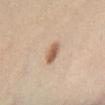Q: Is there a histopathology result?
A: imaged on a skin check; not biopsied
Q: Illumination type?
A: cross-polarized illumination
Q: Patient demographics?
A: female, in their 40s
Q: What is the anatomic site?
A: the leg
Q: How was this image acquired?
A: ~15 mm tile from a whole-body skin photo